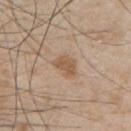workup: no biopsy performed (imaged during a skin exam)
automated metrics: an area of roughly 4.5 mm² and an outline eccentricity of about 0.75 (0 = round, 1 = elongated); an automated nevus-likeness rating near 35 out of 100 and lesion-presence confidence of about 100/100
size: about 3 mm
image source: 15 mm crop, total-body photography
subject: male, aged approximately 50
tile lighting: white-light illumination
body site: the left upper arm